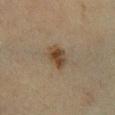Approximately 3 mm at its widest. A female subject in their 40s. Cropped from a whole-body photographic skin survey; the tile spans about 15 mm. This is a cross-polarized tile. Located on the right lower leg.